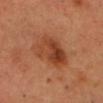Part of a total-body skin-imaging series; this lesion was reviewed on a skin check and was not flagged for biopsy. A close-up tile cropped from a whole-body skin photograph, about 15 mm across. The recorded lesion diameter is about 5.5 mm. Captured under cross-polarized illumination. The lesion is on the head or neck. A male subject about 55 years old.